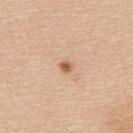• workup · imaged on a skin check; not biopsied
• anatomic site · the back
• lesion diameter · about 1.5 mm
• subject · female, roughly 55 years of age
• image source · 15 mm crop, total-body photography
• image-analysis metrics · a nevus-likeness score of about 90/100 and a detector confidence of about 100 out of 100 that the crop contains a lesion
• illumination · white-light